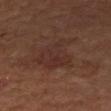Recorded during total-body skin imaging; not selected for excision or biopsy.
A female subject, aged approximately 50.
Cropped from a total-body skin-imaging series; the visible field is about 15 mm.
From the left forearm.
Automated tile analysis of the lesion measured a shape-asymmetry score of about 0.4 (0 = symmetric). It also reported border irregularity of about 4.5 on a 0–10 scale, internal color variation of about 3.5 on a 0–10 scale, and a peripheral color-asymmetry measure near 1.
Measured at roughly 5 mm in maximum diameter.